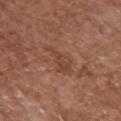Clinical impression: No biopsy was performed on this lesion — it was imaged during a full skin examination and was not determined to be concerning. Clinical summary: A female subject, in their mid- to late 70s. The lesion is on the chest. Cropped from a whole-body photographic skin survey; the tile spans about 15 mm. Longest diameter approximately 4 mm.